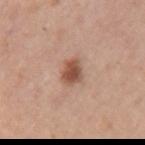biopsy_status: not biopsied; imaged during a skin examination
patient:
  sex: female
  age_approx: 60
image:
  source: total-body photography crop
  field_of_view_mm: 15
lighting: white-light
automated_metrics:
  eccentricity: 0.5
  shape_asymmetry: 0.2
  vs_skin_darker_L: 13.0
  vs_skin_contrast_norm: 9.0
  border_irregularity_0_10: 1.5
  color_variation_0_10: 3.5
  peripheral_color_asymmetry: 1.0
  nevus_likeness_0_100: 95
  lesion_detection_confidence_0_100: 100
site: left upper arm